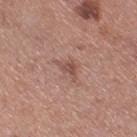Recorded during total-body skin imaging; not selected for excision or biopsy. Imaged with white-light lighting. Measured at roughly 3 mm in maximum diameter. The subject is a female aged 28–32. An algorithmic analysis of the crop reported a shape eccentricity near 0.8 and a symmetry-axis asymmetry near 0.5. The software also gave a border-irregularity rating of about 5/10, a within-lesion color-variation index near 2.5/10, and radial color variation of about 1. The software also gave an automated nevus-likeness rating near 0 out of 100 and a lesion-detection confidence of about 100/100. A region of skin cropped from a whole-body photographic capture, roughly 15 mm wide. From the right thigh.